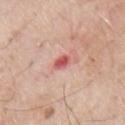The lesion was photographed on a routine skin check and not biopsied; there is no pathology result.